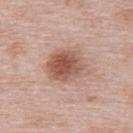| feature | finding |
|---|---|
| biopsy status | catalogued during a skin exam; not biopsied |
| body site | the back |
| diameter | ≈5 mm |
| acquisition | total-body-photography crop, ~15 mm field of view |
| patient | female, aged approximately 65 |